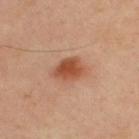Clinical impression: The lesion was tiled from a total-body skin photograph and was not biopsied. Acquisition and patient details: A male patient, aged around 35. A close-up tile cropped from a whole-body skin photograph, about 15 mm across. On the upper back. Longest diameter approximately 4 mm.